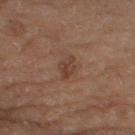- workup: total-body-photography surveillance lesion; no biopsy
- subject: male, roughly 70 years of age
- tile lighting: cross-polarized
- image source: ~15 mm crop, total-body skin-cancer survey
- lesion diameter: ≈3 mm
- automated lesion analysis: a footprint of about 4.5 mm² and a symmetry-axis asymmetry near 0.3
- location: the right thigh